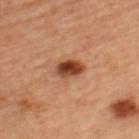Image and clinical context: A female subject, approximately 45 years of age. From the back. A close-up tile cropped from a whole-body skin photograph, about 15 mm across.